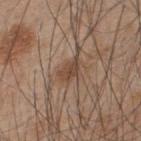Q: Was a biopsy performed?
A: no biopsy performed (imaged during a skin exam)